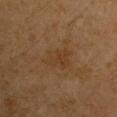This lesion was catalogued during total-body skin photography and was not selected for biopsy. The recorded lesion diameter is about 3.5 mm. Captured under cross-polarized illumination. A 15 mm crop from a total-body photograph taken for skin-cancer surveillance. On the right upper arm. Automated image analysis of the tile measured a nevus-likeness score of about 0/100. A male subject approximately 60 years of age.